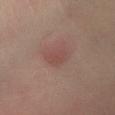Assessment: The lesion was photographed on a routine skin check and not biopsied; there is no pathology result. Acquisition and patient details: From the left lower leg. A roughly 15 mm field-of-view crop from a total-body skin photograph. A female patient aged approximately 55. About 3 mm across. Captured under cross-polarized illumination.